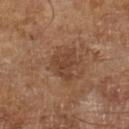No biopsy was performed on this lesion — it was imaged during a full skin examination and was not determined to be concerning.
A male subject, in their mid- to late 60s.
Longest diameter approximately 4 mm.
A lesion tile, about 15 mm wide, cut from a 3D total-body photograph.